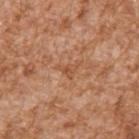No biopsy was performed on this lesion — it was imaged during a full skin examination and was not determined to be concerning.
Cropped from a total-body skin-imaging series; the visible field is about 15 mm.
A male patient, about 45 years old.
From the right upper arm.